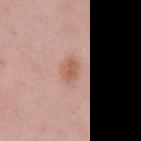notes — catalogued during a skin exam; not biopsied | automated lesion analysis — a normalized lesion–skin contrast near 7.5 | lighting — white-light illumination | site — the arm | image — ~15 mm crop, total-body skin-cancer survey | lesion diameter — about 2.5 mm | patient — female, aged 23 to 27.